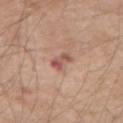Case summary:
– workup: catalogued during a skin exam; not biopsied
– subject: male, roughly 60 years of age
– acquisition: 15 mm crop, total-body photography
– site: the left forearm
– size: ≈3 mm
– lighting: white-light